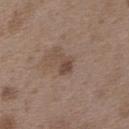Recorded during total-body skin imaging; not selected for excision or biopsy. Captured under white-light illumination. A female subject roughly 35 years of age. The lesion is located on the upper back. Cropped from a whole-body photographic skin survey; the tile spans about 15 mm.